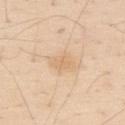{"biopsy_status": "not biopsied; imaged during a skin examination", "patient": {"sex": "male", "age_approx": 80}, "lighting": "white-light", "site": "back", "image": {"source": "total-body photography crop", "field_of_view_mm": 15}, "lesion_size": {"long_diameter_mm_approx": 3.0}}